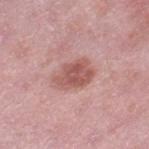Q: Was a biopsy performed?
A: imaged on a skin check; not biopsied
Q: What kind of image is this?
A: 15 mm crop, total-body photography
Q: How large is the lesion?
A: ~4.5 mm (longest diameter)
Q: Lesion location?
A: the left thigh
Q: What lighting was used for the tile?
A: white-light illumination
Q: Automated lesion metrics?
A: a footprint of about 10 mm², an outline eccentricity of about 0.7 (0 = round, 1 = elongated), and two-axis asymmetry of about 0.25; a border-irregularity rating of about 2.5/10, a color-variation rating of about 3.5/10, and radial color variation of about 1; a lesion-detection confidence of about 100/100
Q: What are the patient's age and sex?
A: female, aged around 40